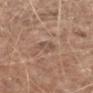- workup — catalogued during a skin exam; not biopsied
- tile lighting — white-light illumination
- acquisition — ~15 mm crop, total-body skin-cancer survey
- patient — male, aged 58 to 62
- lesion size — about 3 mm
- body site — the right forearm